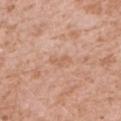  patient:
    sex: female
    age_approx: 40
  lighting: white-light
  image:
    source: total-body photography crop
    field_of_view_mm: 15
  automated_metrics:
    area_mm2_approx: 2.5
    eccentricity: 0.9
    shape_asymmetry: 0.55
    cielab_L: 61
    cielab_a: 22
    cielab_b: 32
    vs_skin_contrast_norm: 5.0
  lesion_size:
    long_diameter_mm_approx: 2.5
  site: arm